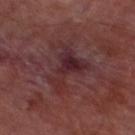A male subject aged 68 to 72.
This image is a 15 mm lesion crop taken from a total-body photograph.
About 6.5 mm across.
From the leg.
Captured under cross-polarized illumination.